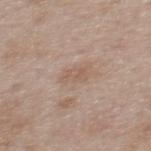• biopsy status — total-body-photography surveillance lesion; no biopsy
• patient — female, aged 38 to 42
• imaging modality — ~15 mm crop, total-body skin-cancer survey
• tile lighting — white-light
• body site — the upper back
• lesion diameter — ~3 mm (longest diameter)
• automated metrics — a lesion–skin lightness drop of about 6 and a lesion-to-skin contrast of about 5 (normalized; higher = more distinct); border irregularity of about 3 on a 0–10 scale, a within-lesion color-variation index near 2/10, and a peripheral color-asymmetry measure near 0.5; a classifier nevus-likeness of about 0/100